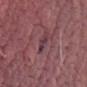biopsy status: imaged on a skin check; not biopsied | image source: ~15 mm tile from a whole-body skin photo | illumination: white-light | subject: male, aged approximately 70 | diameter: ≈3.5 mm | automated metrics: a footprint of about 3.5 mm², an eccentricity of roughly 0.95, and a shape-asymmetry score of about 0.3 (0 = symmetric); a border-irregularity rating of about 4/10, internal color variation of about 1 on a 0–10 scale, and a peripheral color-asymmetry measure near 0; a classifier nevus-likeness of about 0/100 and a lesion-detection confidence of about 85/100 | anatomic site: the left thigh.